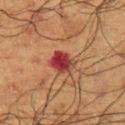* workup · no biopsy performed (imaged during a skin exam)
* size · about 3 mm
* subject · male, aged approximately 60
* image · total-body-photography crop, ~15 mm field of view
* illumination · cross-polarized illumination
* site · the left upper arm